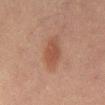| key | value |
|---|---|
| notes | catalogued during a skin exam; not biopsied |
| tile lighting | cross-polarized illumination |
| patient | male, aged 63–67 |
| anatomic site | the mid back |
| TBP lesion metrics | a mean CIELAB color near L≈39 a*≈19 b*≈25 and about 7 CIELAB-L* units darker than the surrounding skin; a border-irregularity rating of about 1.5/10 and radial color variation of about 1 |
| image | 15 mm crop, total-body photography |